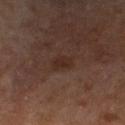| feature | finding |
|---|---|
| anatomic site | the left lower leg |
| image | total-body-photography crop, ~15 mm field of view |
| size | ~3 mm (longest diameter) |
| illumination | cross-polarized illumination |
| TBP lesion metrics | an eccentricity of roughly 0.8 and a shape-asymmetry score of about 0.25 (0 = symmetric); a lesion color around L≈23 a*≈15 b*≈20 in CIELAB; border irregularity of about 3 on a 0–10 scale, a color-variation rating of about 1.5/10, and a peripheral color-asymmetry measure near 0.5 |
| subject | female, aged approximately 60 |